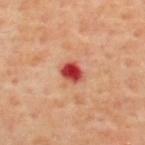workup = no biopsy performed (imaged during a skin exam); subject = male, in their mid-60s; acquisition = ~15 mm tile from a whole-body skin photo; size = ~2.5 mm (longest diameter); illumination = cross-polarized illumination; site = the upper back.